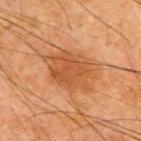Clinical impression:
Recorded during total-body skin imaging; not selected for excision or biopsy.
Background:
Imaged with cross-polarized lighting. Located on the back. A 15 mm crop from a total-body photograph taken for skin-cancer surveillance. Approximately 6.5 mm at its widest. A male patient, aged 63 to 67.